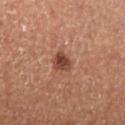Captured during whole-body skin photography for melanoma surveillance; the lesion was not biopsied. The recorded lesion diameter is about 2.5 mm. From the right lower leg. A 15 mm close-up tile from a total-body photography series done for melanoma screening. A male patient aged 58–62.